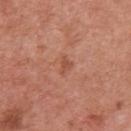The lesion was tiled from a total-body skin photograph and was not biopsied.
The lesion is located on the arm.
The subject is a female about 50 years old.
The total-body-photography lesion software estimated a lesion color around L≈52 a*≈26 b*≈32 in CIELAB, a lesion–skin lightness drop of about 7, and a normalized lesion–skin contrast near 5.5. The software also gave a border-irregularity rating of about 4.5/10, a color-variation rating of about 1/10, and a peripheral color-asymmetry measure near 0. It also reported a classifier nevus-likeness of about 0/100 and lesion-presence confidence of about 100/100.
A 15 mm close-up extracted from a 3D total-body photography capture.
The recorded lesion diameter is about 2.5 mm.